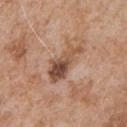Notes:
• subject: male, aged 63 to 67
• body site: the front of the torso
• image source: ~15 mm crop, total-body skin-cancer survey
• diameter: ~5.5 mm (longest diameter)
• automated lesion analysis: a lesion area of about 11 mm² and a shape eccentricity near 0.9; a lesion color around L≈51 a*≈20 b*≈30 in CIELAB, about 12 CIELAB-L* units darker than the surrounding skin, and a normalized lesion–skin contrast near 8.5; a border-irregularity rating of about 5/10; a nevus-likeness score of about 25/100 and lesion-presence confidence of about 100/100
• tile lighting: white-light illumination